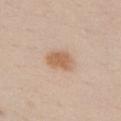Clinical impression:
The lesion was photographed on a routine skin check and not biopsied; there is no pathology result.
Acquisition and patient details:
Approximately 3.5 mm at its widest. A female patient aged 23–27. This image is a 15 mm lesion crop taken from a total-body photograph. On the chest.